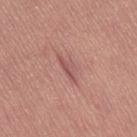Findings:
– biopsy status: no biopsy performed (imaged during a skin exam)
– imaging modality: total-body-photography crop, ~15 mm field of view
– subject: female, aged around 55
– location: the right thigh